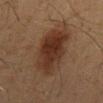Case summary:
– workup: imaged on a skin check; not biopsied
– body site: the mid back
– subject: male, aged 58 to 62
– image source: total-body-photography crop, ~15 mm field of view